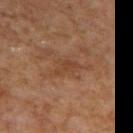Recorded during total-body skin imaging; not selected for excision or biopsy.
The total-body-photography lesion software estimated a footprint of about 3 mm² and a shape-asymmetry score of about 0.55 (0 = symmetric).
On the upper back.
A female patient, about 60 years old.
About 2.5 mm across.
Imaged with cross-polarized lighting.
A 15 mm crop from a total-body photograph taken for skin-cancer surveillance.